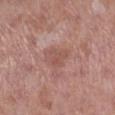{"biopsy_status": "not biopsied; imaged during a skin examination", "image": {"source": "total-body photography crop", "field_of_view_mm": 15}, "automated_metrics": {"cielab_L": 52, "cielab_a": 23, "cielab_b": 25, "color_variation_0_10": 1.5}, "patient": {"sex": "female", "age_approx": 50}, "site": "right lower leg", "lesion_size": {"long_diameter_mm_approx": 2.5}}